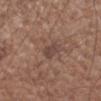From the left forearm.
A 15 mm crop from a total-body photograph taken for skin-cancer surveillance.
A male patient, roughly 55 years of age.